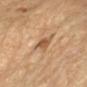This lesion was catalogued during total-body skin photography and was not selected for biopsy. A female subject, roughly 60 years of age. The total-body-photography lesion software estimated a footprint of about 4.5 mm² and a shape eccentricity near 0.75. The software also gave a mean CIELAB color near L≈56 a*≈20 b*≈36. The analysis additionally found a border-irregularity rating of about 3/10 and a within-lesion color-variation index near 2.5/10. This image is a 15 mm lesion crop taken from a total-body photograph. Captured under cross-polarized illumination. From the left forearm.